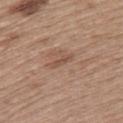Impression:
Imaged during a routine full-body skin examination; the lesion was not biopsied and no histopathology is available.
Clinical summary:
Automated image analysis of the tile measured an average lesion color of about L≈51 a*≈19 b*≈28 (CIELAB) and a normalized lesion–skin contrast near 6. And it measured a border-irregularity index near 3.5/10 and radial color variation of about 0. And it measured a nevus-likeness score of about 0/100 and a detector confidence of about 100 out of 100 that the crop contains a lesion. This is a white-light tile. A lesion tile, about 15 mm wide, cut from a 3D total-body photograph. The subject is a female approximately 60 years of age. From the upper back. Measured at roughly 3 mm in maximum diameter.